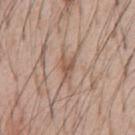Clinical impression:
The lesion was tiled from a total-body skin photograph and was not biopsied.
Acquisition and patient details:
The lesion is located on the chest. A 15 mm close-up tile from a total-body photography series done for melanoma screening. The recorded lesion diameter is about 2.5 mm. A male patient, aged 53 to 57.